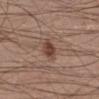Notes:
• notes: catalogued during a skin exam; not biopsied
• body site: the right lower leg
• lighting: white-light
• patient: male, aged approximately 30
• lesion diameter: about 2.5 mm
• acquisition: 15 mm crop, total-body photography